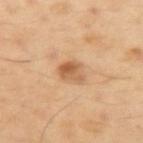{
  "biopsy_status": "not biopsied; imaged during a skin examination",
  "image": {
    "source": "total-body photography crop",
    "field_of_view_mm": 15
  },
  "site": "arm",
  "lesion_size": {
    "long_diameter_mm_approx": 3.0
  },
  "lighting": "cross-polarized",
  "patient": {
    "sex": "male",
    "age_approx": 50
  }
}